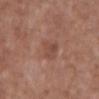Imaged with white-light lighting.
Approximately 3 mm at its widest.
From the abdomen.
A region of skin cropped from a whole-body photographic capture, roughly 15 mm wide.
A male subject roughly 75 years of age.